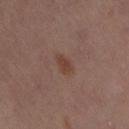| feature | finding |
|---|---|
| follow-up | imaged on a skin check; not biopsied |
| body site | the left thigh |
| image | ~15 mm tile from a whole-body skin photo |
| subject | female, aged around 50 |
| lesion diameter | ≈3 mm |